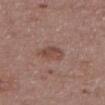Q: Was a biopsy performed?
A: catalogued during a skin exam; not biopsied
Q: Lesion size?
A: ≈3 mm
Q: What is the imaging modality?
A: ~15 mm crop, total-body skin-cancer survey
Q: Lesion location?
A: the upper back
Q: Patient demographics?
A: female, in their mid- to late 60s